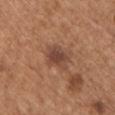Assessment: Captured during whole-body skin photography for melanoma surveillance; the lesion was not biopsied. Acquisition and patient details: Automated tile analysis of the lesion measured an average lesion color of about L≈45 a*≈21 b*≈28 (CIELAB). And it measured a border-irregularity index near 3.5/10 and a peripheral color-asymmetry measure near 1.5. It also reported a classifier nevus-likeness of about 10/100 and a lesion-detection confidence of about 100/100. Cropped from a total-body skin-imaging series; the visible field is about 15 mm. The tile uses white-light illumination. Longest diameter approximately 3.5 mm. The lesion is on the chest. A male patient roughly 65 years of age.